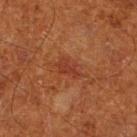Case summary:
• notes · total-body-photography surveillance lesion; no biopsy
• imaging modality · total-body-photography crop, ~15 mm field of view
• subject · male, in their mid-60s
• location · the leg